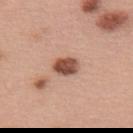The lesion was tiled from a total-body skin photograph and was not biopsied.
A 15 mm close-up extracted from a 3D total-body photography capture.
A female patient, aged 58–62.
The lesion's longest dimension is about 3 mm.
The total-body-photography lesion software estimated a footprint of about 5.5 mm², an eccentricity of roughly 0.75, and two-axis asymmetry of about 0.15. The analysis additionally found a border-irregularity index near 1.5/10 and a within-lesion color-variation index near 4/10.
The lesion is on the upper back.